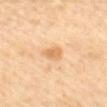Captured during whole-body skin photography for melanoma surveillance; the lesion was not biopsied. A close-up tile cropped from a whole-body skin photograph, about 15 mm across. A female patient, aged around 60. The lesion's longest dimension is about 2.5 mm. Located on the mid back.